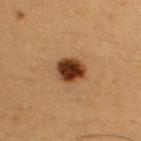Findings:
– biopsy status · no biopsy performed (imaged during a skin exam)
– site · the chest
– image · 15 mm crop, total-body photography
– subject · male, roughly 50 years of age